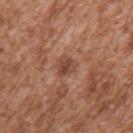lesion size = about 2.5 mm; body site = the left upper arm; illumination = white-light illumination; image = 15 mm crop, total-body photography; subject = male, in their mid- to late 40s.